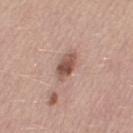Notes:
– workup — imaged on a skin check; not biopsied
– site — the right thigh
– automated lesion analysis — a lesion color around L≈52 a*≈21 b*≈25 in CIELAB and roughly 14 lightness units darker than nearby skin; border irregularity of about 2.5 on a 0–10 scale and a peripheral color-asymmetry measure near 1.5
– illumination — white-light illumination
– size — ~3.5 mm (longest diameter)
– patient — female, roughly 45 years of age
– image — ~15 mm crop, total-body skin-cancer survey